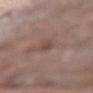No biopsy was performed on this lesion — it was imaged during a full skin examination and was not determined to be concerning. A female subject, aged around 70. Measured at roughly 2.5 mm in maximum diameter. Cropped from a whole-body photographic skin survey; the tile spans about 15 mm. On the left forearm.